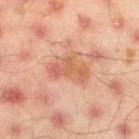Part of a total-body skin-imaging series; this lesion was reviewed on a skin check and was not flagged for biopsy.
A male subject, roughly 45 years of age.
A close-up tile cropped from a whole-body skin photograph, about 15 mm across.
The total-body-photography lesion software estimated an area of roughly 9.5 mm², an outline eccentricity of about 0.8 (0 = round, 1 = elongated), and a symmetry-axis asymmetry near 0.45. And it measured a mean CIELAB color near L≈61 a*≈26 b*≈34, roughly 9 lightness units darker than nearby skin, and a normalized lesion–skin contrast near 6.5. The analysis additionally found a border-irregularity index near 5/10, internal color variation of about 6.5 on a 0–10 scale, and radial color variation of about 2.5.
Located on the left thigh.